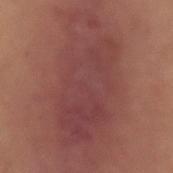notes: total-body-photography surveillance lesion; no biopsy | size: about 13 mm | image source: 15 mm crop, total-body photography | TBP lesion metrics: a lesion area of about 60 mm² and two-axis asymmetry of about 0.25 | tile lighting: cross-polarized | anatomic site: the arm | patient: male, approximately 55 years of age.